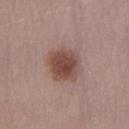The lesion was tiled from a total-body skin photograph and was not biopsied. A female patient, approximately 50 years of age. On the right lower leg. Imaged with white-light lighting. Approximately 4 mm at its widest. A region of skin cropped from a whole-body photographic capture, roughly 15 mm wide.